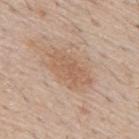Impression:
Part of a total-body skin-imaging series; this lesion was reviewed on a skin check and was not flagged for biopsy.
Context:
A region of skin cropped from a whole-body photographic capture, roughly 15 mm wide. A male patient aged 53 to 57. Located on the upper back.